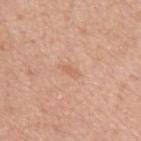{"biopsy_status": "not biopsied; imaged during a skin examination", "site": "arm", "image": {"source": "total-body photography crop", "field_of_view_mm": 15}, "lighting": "white-light", "lesion_size": {"long_diameter_mm_approx": 2.5}, "patient": {"sex": "male", "age_approx": 50}}